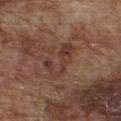The lesion was photographed on a routine skin check and not biopsied; there is no pathology result.
Located on the front of the torso.
Cropped from a whole-body photographic skin survey; the tile spans about 15 mm.
The recorded lesion diameter is about 4.5 mm.
The patient is a male about 75 years old.